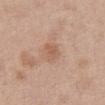| field | value |
|---|---|
| workup | total-body-photography surveillance lesion; no biopsy |
| imaging modality | total-body-photography crop, ~15 mm field of view |
| location | the front of the torso |
| subject | female, aged 38–42 |